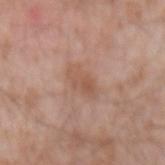Recorded during total-body skin imaging; not selected for excision or biopsy.
Captured under white-light illumination.
The lesion is on the right forearm.
A region of skin cropped from a whole-body photographic capture, roughly 15 mm wide.
The patient is a male in their 60s.